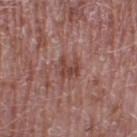Impression:
Captured during whole-body skin photography for melanoma surveillance; the lesion was not biopsied.
Background:
Located on the right thigh. A male subject, aged around 60. A 15 mm close-up extracted from a 3D total-body photography capture.